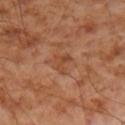Imaged during a routine full-body skin examination; the lesion was not biopsied and no histopathology is available. This is a cross-polarized tile. A 15 mm close-up extracted from a 3D total-body photography capture. A male patient in their mid-50s. The lesion is on the right forearm.